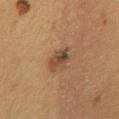No biopsy was performed on this lesion — it was imaged during a full skin examination and was not determined to be concerning.
A lesion tile, about 15 mm wide, cut from a 3D total-body photograph.
From the mid back.
The recorded lesion diameter is about 3.5 mm.
The subject is a female approximately 50 years of age.